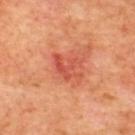Imaged during a routine full-body skin examination; the lesion was not biopsied and no histopathology is available. The lesion is on the upper back. A subject in their mid-60s. Measured at roughly 4 mm in maximum diameter. A 15 mm close-up tile from a total-body photography series done for melanoma screening. Imaged with cross-polarized lighting.